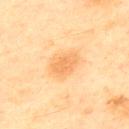Impression:
Imaged during a routine full-body skin examination; the lesion was not biopsied and no histopathology is available.
Image and clinical context:
From the back. A male subject, aged 43 to 47. A 15 mm close-up extracted from a 3D total-body photography capture. Automated tile analysis of the lesion measured a footprint of about 7.5 mm², an eccentricity of roughly 0.7, and a symmetry-axis asymmetry near 0.25. The analysis additionally found a within-lesion color-variation index near 2/10 and radial color variation of about 0.5. Imaged with cross-polarized lighting.